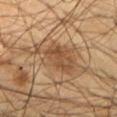Q: Is there a histopathology result?
A: catalogued during a skin exam; not biopsied
Q: What is the anatomic site?
A: the front of the torso
Q: What kind of image is this?
A: total-body-photography crop, ~15 mm field of view
Q: What are the patient's age and sex?
A: male, about 55 years old